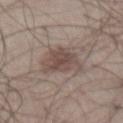Assessment:
The lesion was tiled from a total-body skin photograph and was not biopsied.
Clinical summary:
About 5.5 mm across. Cropped from a whole-body photographic skin survey; the tile spans about 15 mm. Automated image analysis of the tile measured a footprint of about 12 mm² and a symmetry-axis asymmetry near 0.35. The analysis additionally found a detector confidence of about 100 out of 100 that the crop contains a lesion. Captured under white-light illumination. A male patient, approximately 55 years of age. Located on the abdomen.